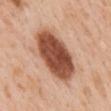workup: no biopsy performed (imaged during a skin exam) | lesion size: ~7 mm (longest diameter) | TBP lesion metrics: an eccentricity of roughly 0.8; a mean CIELAB color near L≈50 a*≈25 b*≈32, a lesion–skin lightness drop of about 20, and a lesion-to-skin contrast of about 13 (normalized; higher = more distinct); a border-irregularity rating of about 1.5/10; a classifier nevus-likeness of about 60/100 | tile lighting: white-light | body site: the mid back | image source: 15 mm crop, total-body photography | subject: female, aged approximately 50.